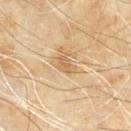Q: Was a biopsy performed?
A: catalogued during a skin exam; not biopsied
Q: Illumination type?
A: cross-polarized illumination
Q: What is the anatomic site?
A: the mid back
Q: How was this image acquired?
A: 15 mm crop, total-body photography
Q: How large is the lesion?
A: ~2.5 mm (longest diameter)
Q: What are the patient's age and sex?
A: male, aged approximately 60